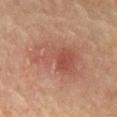Q: Is there a histopathology result?
A: imaged on a skin check; not biopsied
Q: Lesion size?
A: ≈7 mm
Q: How was this image acquired?
A: 15 mm crop, total-body photography
Q: Who is the patient?
A: male, approximately 65 years of age
Q: How was the tile lit?
A: cross-polarized
Q: What is the anatomic site?
A: the chest